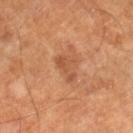Clinical impression: No biopsy was performed on this lesion — it was imaged during a full skin examination and was not determined to be concerning. Background: A male patient approximately 60 years of age. Cropped from a total-body skin-imaging series; the visible field is about 15 mm. On the right lower leg.